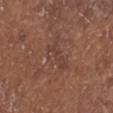follow-up — catalogued during a skin exam; not biopsied
site — the head or neck
image — total-body-photography crop, ~15 mm field of view
illumination — white-light
subject — male, approximately 80 years of age
image-analysis metrics — a footprint of about 5.5 mm² and two-axis asymmetry of about 0.4; a border-irregularity index near 5.5/10 and radial color variation of about 0.5
diameter — ≈4 mm